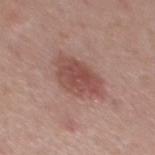Impression: The lesion was tiled from a total-body skin photograph and was not biopsied. Clinical summary: On the mid back. A male subject, aged 53–57. Cropped from a whole-body photographic skin survey; the tile spans about 15 mm. Automated image analysis of the tile measured a mean CIELAB color near L≈49 a*≈23 b*≈24 and roughly 10 lightness units darker than nearby skin. The software also gave a within-lesion color-variation index near 4/10 and radial color variation of about 1.5.